Cropped from a total-body skin-imaging series; the visible field is about 15 mm. The lesion is located on the chest. A male patient roughly 70 years of age: 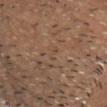automated_metrics:
  eccentricity: 0.95
  shape_asymmetry: 0.45
  cielab_L: 47
  cielab_a: 16
  cielab_b: 29
  vs_skin_contrast_norm: 3.5
  border_irregularity_0_10: 5.5
  color_variation_0_10: 0.0
  peripheral_color_asymmetry: 0.0
lighting: white-light
lesion_size:
  long_diameter_mm_approx: 2.5
diagnosis:
  histopathology: verruca
  malignancy: benign
  taxonomic_path:
    - Benign
    - Inflammatory or infectious diseases
    - Verruca